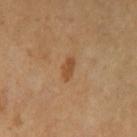No biopsy was performed on this lesion — it was imaged during a full skin examination and was not determined to be concerning. The lesion is on the left thigh. The lesion's longest dimension is about 2.5 mm. A 15 mm close-up extracted from a 3D total-body photography capture. A female subject, aged around 55. Captured under cross-polarized illumination.